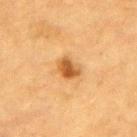Notes:
* workup — imaged on a skin check; not biopsied
* automated metrics — an outline eccentricity of about 0.7 (0 = round, 1 = elongated) and a symmetry-axis asymmetry near 0.2; a lesion color around L≈51 a*≈22 b*≈41 in CIELAB, roughly 12 lightness units darker than nearby skin, and a normalized border contrast of about 9
* body site — the back
* diameter — about 3 mm
* patient — male, aged around 85 or older
* image source — ~15 mm tile from a whole-body skin photo
* illumination — cross-polarized